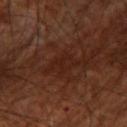Impression: Imaged during a routine full-body skin examination; the lesion was not biopsied and no histopathology is available. Background: The lesion-visualizer software estimated a lesion area of about 5.5 mm², a shape eccentricity near 0.85, and a shape-asymmetry score of about 0.5 (0 = symmetric). It also reported a color-variation rating of about 2/10. And it measured a nevus-likeness score of about 0/100 and lesion-presence confidence of about 80/100. A lesion tile, about 15 mm wide, cut from a 3D total-body photograph. Approximately 4 mm at its widest. Captured under cross-polarized illumination. The lesion is located on the right thigh. A male subject approximately 70 years of age.